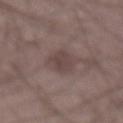Part of a total-body skin-imaging series; this lesion was reviewed on a skin check and was not flagged for biopsy.
A 15 mm crop from a total-body photograph taken for skin-cancer surveillance.
Imaged with white-light lighting.
The lesion is located on the front of the torso.
The patient is a male about 60 years old.